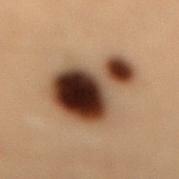Part of a total-body skin-imaging series; this lesion was reviewed on a skin check and was not flagged for biopsy. A male patient, approximately 55 years of age. Cropped from a total-body skin-imaging series; the visible field is about 15 mm. Located on the back.